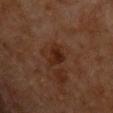Captured during whole-body skin photography for melanoma surveillance; the lesion was not biopsied.
An algorithmic analysis of the crop reported a lesion area of about 5 mm², an outline eccentricity of about 0.8 (0 = round, 1 = elongated), and a symmetry-axis asymmetry near 0.3.
The subject is a male in their mid-60s.
On the front of the torso.
Cropped from a whole-body photographic skin survey; the tile spans about 15 mm.
Approximately 3.5 mm at its widest.
Captured under cross-polarized illumination.